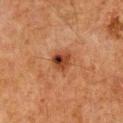The lesion was photographed on a routine skin check and not biopsied; there is no pathology result. This image is a 15 mm lesion crop taken from a total-body photograph. Approximately 2.5 mm at its widest. Automated tile analysis of the lesion measured a lesion area of about 4.5 mm², a shape eccentricity near 0.25, and two-axis asymmetry of about 0.2. It also reported an average lesion color of about L≈33 a*≈23 b*≈29 (CIELAB) and a normalized border contrast of about 10. The software also gave internal color variation of about 9 on a 0–10 scale and radial color variation of about 4. And it measured a classifier nevus-likeness of about 90/100 and a lesion-detection confidence of about 100/100. The tile uses cross-polarized illumination. The lesion is located on the chest. The subject is a male aged 78–82.